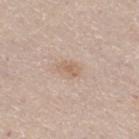Background: A region of skin cropped from a whole-body photographic capture, roughly 15 mm wide. The recorded lesion diameter is about 3 mm. The lesion is located on the right thigh. A female patient approximately 45 years of age.